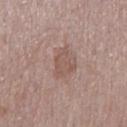Captured during whole-body skin photography for melanoma surveillance; the lesion was not biopsied. A male subject, about 70 years old. The lesion is on the mid back. A close-up tile cropped from a whole-body skin photograph, about 15 mm across. Captured under white-light illumination. Automated tile analysis of the lesion measured a lesion area of about 6 mm² and a shape-asymmetry score of about 0.35 (0 = symmetric). It also reported a lesion color around L≈53 a*≈18 b*≈23 in CIELAB, a lesion–skin lightness drop of about 7, and a normalized border contrast of about 5.5.